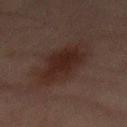Part of a total-body skin-imaging series; this lesion was reviewed on a skin check and was not flagged for biopsy.
A close-up tile cropped from a whole-body skin photograph, about 15 mm across.
Captured under cross-polarized illumination.
The lesion is on the abdomen.
The patient is a male about 65 years old.